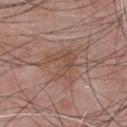Part of a total-body skin-imaging series; this lesion was reviewed on a skin check and was not flagged for biopsy. Cropped from a total-body skin-imaging series; the visible field is about 15 mm. From the chest. The total-body-photography lesion software estimated a lesion area of about 8.5 mm², an outline eccentricity of about 0.3 (0 = round, 1 = elongated), and a symmetry-axis asymmetry near 0.5. And it measured a classifier nevus-likeness of about 0/100. A male patient, in their mid-50s. The tile uses white-light illumination. The lesion's longest dimension is about 4 mm.